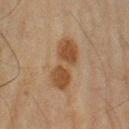The lesion was tiled from a total-body skin photograph and was not biopsied.
Located on the left upper arm.
Automated tile analysis of the lesion measured a footprint of about 14 mm², an outline eccentricity of about 0.9 (0 = round, 1 = elongated), and a symmetry-axis asymmetry near 0.2. The analysis additionally found roughly 8 lightness units darker than nearby skin and a lesion-to-skin contrast of about 8 (normalized; higher = more distinct). It also reported a nevus-likeness score of about 65/100 and a lesion-detection confidence of about 100/100.
Captured under cross-polarized illumination.
A male subject approximately 65 years of age.
A roughly 15 mm field-of-view crop from a total-body skin photograph.